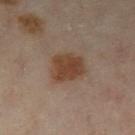  biopsy_status: not biopsied; imaged during a skin examination
  site: leg
  image:
    source: total-body photography crop
    field_of_view_mm: 15
  patient:
    sex: female
    age_approx: 60